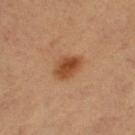Impression:
Captured during whole-body skin photography for melanoma surveillance; the lesion was not biopsied.
Image and clinical context:
A lesion tile, about 15 mm wide, cut from a 3D total-body photograph. The patient is a female aged 58 to 62. From the right thigh.